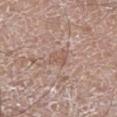This lesion was catalogued during total-body skin photography and was not selected for biopsy.
A close-up tile cropped from a whole-body skin photograph, about 15 mm across.
The lesion is located on the right lower leg.
The subject is a male roughly 60 years of age.